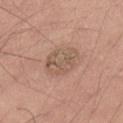| feature | finding |
|---|---|
| notes | imaged on a skin check; not biopsied |
| patient | male, roughly 25 years of age |
| imaging modality | ~15 mm tile from a whole-body skin photo |
| tile lighting | white-light |
| size | ≈4 mm |
| body site | the left upper arm |
| automated metrics | a lesion area of about 12 mm², a shape eccentricity near 0.4, and a symmetry-axis asymmetry near 0.15; border irregularity of about 1.5 on a 0–10 scale; a nevus-likeness score of about 0/100 and lesion-presence confidence of about 100/100 |